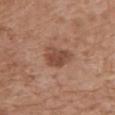<case>
  <biopsy_status>not biopsied; imaged during a skin examination</biopsy_status>
  <image>
    <source>total-body photography crop</source>
    <field_of_view_mm>15</field_of_view_mm>
  </image>
  <automated_metrics>
    <area_mm2_approx>8.0</area_mm2_approx>
    <shape_asymmetry>0.3</shape_asymmetry>
    <cielab_L>47</cielab_L>
    <cielab_a>22</cielab_a>
    <cielab_b>29</cielab_b>
    <border_irregularity_0_10>3.0</border_irregularity_0_10>
    <color_variation_0_10>3.0</color_variation_0_10>
    <peripheral_color_asymmetry>1.0</peripheral_color_asymmetry>
  </automated_metrics>
  <lesion_size>
    <long_diameter_mm_approx>4.0</long_diameter_mm_approx>
  </lesion_size>
  <site>mid back</site>
  <patient>
    <sex>female</sex>
    <age_approx>75</age_approx>
  </patient>
</case>